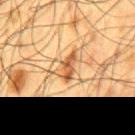Assessment: No biopsy was performed on this lesion — it was imaged during a full skin examination and was not determined to be concerning. Image and clinical context: The lesion is on the mid back. A male patient aged approximately 60. This is a cross-polarized tile. A 15 mm crop from a total-body photograph taken for skin-cancer surveillance.